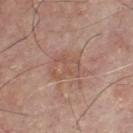The lesion was tiled from a total-body skin photograph and was not biopsied. Located on the chest. The patient is a male approximately 80 years of age. The total-body-photography lesion software estimated an average lesion color of about L≈54 a*≈20 b*≈26 (CIELAB) and a normalized lesion–skin contrast near 4.5. The software also gave a border-irregularity index near 3.5/10, a color-variation rating of about 3.5/10, and peripheral color asymmetry of about 1. A 15 mm crop from a total-body photograph taken for skin-cancer surveillance. Captured under white-light illumination. The lesion's longest dimension is about 5 mm.